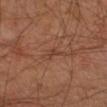Case summary:
* workup — no biopsy performed (imaged during a skin exam)
* acquisition — total-body-photography crop, ~15 mm field of view
* TBP lesion metrics — a lesion area of about 2.5 mm², an eccentricity of roughly 0.9, and two-axis asymmetry of about 0.55; about 6 CIELAB-L* units darker than the surrounding skin; a classifier nevus-likeness of about 0/100 and a detector confidence of about 50 out of 100 that the crop contains a lesion
* patient — male, approximately 55 years of age
* site — the arm